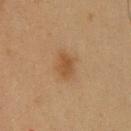| field | value |
|---|---|
| workup | catalogued during a skin exam; not biopsied |
| patient | male, roughly 35 years of age |
| site | the upper back |
| imaging modality | total-body-photography crop, ~15 mm field of view |